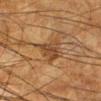{
  "biopsy_status": "not biopsied; imaged during a skin examination",
  "image": {
    "source": "total-body photography crop",
    "field_of_view_mm": 15
  },
  "patient": {
    "sex": "male",
    "age_approx": 65
  },
  "site": "leg"
}